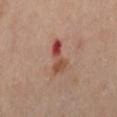<record>
  <patient>
    <sex>female</sex>
    <age_approx>55</age_approx>
  </patient>
  <image>
    <source>total-body photography crop</source>
    <field_of_view_mm>15</field_of_view_mm>
  </image>
  <site>left thigh</site>
  <lesion_size>
    <long_diameter_mm_approx>4.5</long_diameter_mm_approx>
  </lesion_size>
  <automated_metrics>
    <area_mm2_approx>7.0</area_mm2_approx>
    <shape_asymmetry>0.45</shape_asymmetry>
    <border_irregularity_0_10>5.5</border_irregularity_0_10>
    <color_variation_0_10>10.0</color_variation_0_10>
  </automated_metrics>
  <lighting>cross-polarized</lighting>
</record>